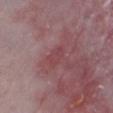Part of a total-body skin-imaging series; this lesion was reviewed on a skin check and was not flagged for biopsy.
Approximately 3 mm at its widest.
Automated image analysis of the tile measured an area of roughly 3.5 mm², an outline eccentricity of about 0.85 (0 = round, 1 = elongated), and two-axis asymmetry of about 0.3. It also reported an average lesion color of about L≈44 a*≈27 b*≈18 (CIELAB), roughly 5 lightness units darker than nearby skin, and a normalized border contrast of about 4.5. It also reported a within-lesion color-variation index near 1/10 and peripheral color asymmetry of about 0.5. And it measured a classifier nevus-likeness of about 0/100 and a lesion-detection confidence of about 60/100.
The patient is a male approximately 65 years of age.
Cropped from a total-body skin-imaging series; the visible field is about 15 mm.
This is a white-light tile.
From the right lower leg.